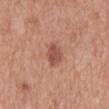Imaged during a routine full-body skin examination; the lesion was not biopsied and no histopathology is available. A close-up tile cropped from a whole-body skin photograph, about 15 mm across. The subject is a male aged approximately 70. Captured under white-light illumination. The lesion is located on the mid back. The lesion's longest dimension is about 3.5 mm.